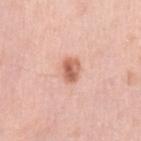No biopsy was performed on this lesion — it was imaged during a full skin examination and was not determined to be concerning.
A female subject, aged approximately 40.
This is a white-light tile.
The lesion is on the left upper arm.
About 3 mm across.
A lesion tile, about 15 mm wide, cut from a 3D total-body photograph.
The lesion-visualizer software estimated a border-irregularity rating of about 2/10, internal color variation of about 5 on a 0–10 scale, and radial color variation of about 2. The analysis additionally found an automated nevus-likeness rating near 95 out of 100 and lesion-presence confidence of about 100/100.